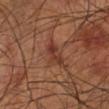Clinical impression: Recorded during total-body skin imaging; not selected for excision or biopsy. Acquisition and patient details: The lesion is located on the leg. The total-body-photography lesion software estimated a footprint of about 4.5 mm² and a shape-asymmetry score of about 0.35 (0 = symmetric). And it measured a classifier nevus-likeness of about 0/100 and a detector confidence of about 95 out of 100 that the crop contains a lesion. The lesion's longest dimension is about 3.5 mm. Imaged with cross-polarized lighting. A male patient aged approximately 70. A close-up tile cropped from a whole-body skin photograph, about 15 mm across.